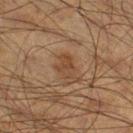| key | value |
|---|---|
| notes | no biopsy performed (imaged during a skin exam) |
| acquisition | 15 mm crop, total-body photography |
| location | the right thigh |
| patient | male, in their 60s |
| lighting | cross-polarized illumination |